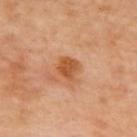{
  "image": {
    "source": "total-body photography crop",
    "field_of_view_mm": 15
  },
  "site": "upper back",
  "lesion_size": {
    "long_diameter_mm_approx": 2.5
  }
}